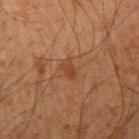Clinical impression: The lesion was photographed on a routine skin check and not biopsied; there is no pathology result. Image and clinical context: The lesion is on the left upper arm. The subject is a male aged approximately 35. The tile uses cross-polarized illumination. A region of skin cropped from a whole-body photographic capture, roughly 15 mm wide.